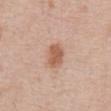* workup: no biopsy performed (imaged during a skin exam)
* lesion size: ~3 mm (longest diameter)
* subject: male, approximately 70 years of age
* body site: the chest
* imaging modality: 15 mm crop, total-body photography